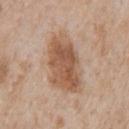No biopsy was performed on this lesion — it was imaged during a full skin examination and was not determined to be concerning.
Imaged with white-light lighting.
From the chest.
Measured at roughly 7 mm in maximum diameter.
A male subject about 65 years old.
A 15 mm crop from a total-body photograph taken for skin-cancer surveillance.
Automated image analysis of the tile measured an area of roughly 22 mm² and an outline eccentricity of about 0.85 (0 = round, 1 = elongated).